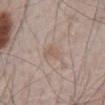Recorded during total-body skin imaging; not selected for excision or biopsy. The patient is a male aged approximately 65. From the abdomen. Captured under white-light illumination. Automated image analysis of the tile measured an outline eccentricity of about 0.75 (0 = round, 1 = elongated) and a shape-asymmetry score of about 0.3 (0 = symmetric). The software also gave a lesion color around L≈57 a*≈15 b*≈25 in CIELAB. It also reported a classifier nevus-likeness of about 0/100 and lesion-presence confidence of about 100/100. Longest diameter approximately 2.5 mm. Cropped from a total-body skin-imaging series; the visible field is about 15 mm.